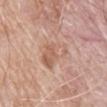patient: male, about 80 years old
lesion size: ≈5 mm
image source: ~15 mm tile from a whole-body skin photo
anatomic site: the mid back
image-analysis metrics: an eccentricity of roughly 0.8 and a shape-asymmetry score of about 0.4 (0 = symmetric); an average lesion color of about L≈61 a*≈20 b*≈29 (CIELAB) and a lesion–skin lightness drop of about 7; an automated nevus-likeness rating near 0 out of 100 and lesion-presence confidence of about 100/100
tile lighting: white-light